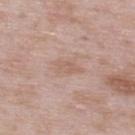Findings:
• follow-up · imaged on a skin check; not biopsied
• automated metrics · a lesion area of about 4 mm², an eccentricity of roughly 0.8, and a shape-asymmetry score of about 0.3 (0 = symmetric); a mean CIELAB color near L≈60 a*≈18 b*≈26, about 6 CIELAB-L* units darker than the surrounding skin, and a normalized border contrast of about 5; a classifier nevus-likeness of about 0/100 and a lesion-detection confidence of about 100/100
• tile lighting · white-light
• lesion diameter · ≈3 mm
• site · the upper back
• acquisition · 15 mm crop, total-body photography
• patient · male, aged 53 to 57